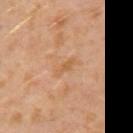Clinical impression: Imaged during a routine full-body skin examination; the lesion was not biopsied and no histopathology is available. Image and clinical context: The subject is a male aged around 30. Located on the left upper arm. A region of skin cropped from a whole-body photographic capture, roughly 15 mm wide.